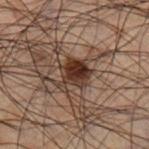{"biopsy_status": "not biopsied; imaged during a skin examination", "lesion_size": {"long_diameter_mm_approx": 4.0}, "image": {"source": "total-body photography crop", "field_of_view_mm": 15}, "lighting": "cross-polarized", "site": "leg", "patient": {"sex": "male", "age_approx": 50}}